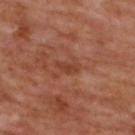Imaged during a routine full-body skin examination; the lesion was not biopsied and no histopathology is available. Imaged with cross-polarized lighting. The total-body-photography lesion software estimated a mean CIELAB color near L≈39 a*≈24 b*≈31, roughly 6 lightness units darker than nearby skin, and a normalized lesion–skin contrast near 6. The analysis additionally found a color-variation rating of about 1/10. The lesion is on the upper back. The patient is a male aged approximately 65. A close-up tile cropped from a whole-body skin photograph, about 15 mm across. About 3 mm across.